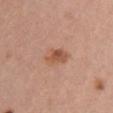Q: Was this lesion biopsied?
A: total-body-photography surveillance lesion; no biopsy
Q: Lesion location?
A: the chest
Q: How was this image acquired?
A: 15 mm crop, total-body photography
Q: Lesion size?
A: ~3.5 mm (longest diameter)
Q: Who is the patient?
A: female, roughly 20 years of age
Q: How was the tile lit?
A: white-light